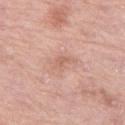The lesion was tiled from a total-body skin photograph and was not biopsied.
From the leg.
This is a white-light tile.
Automated image analysis of the tile measured a classifier nevus-likeness of about 0/100 and a lesion-detection confidence of about 100/100.
Cropped from a whole-body photographic skin survey; the tile spans about 15 mm.
Approximately 3 mm at its widest.
A female patient, in their 70s.